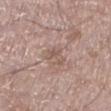No biopsy was performed on this lesion — it was imaged during a full skin examination and was not determined to be concerning. From the left lower leg. The recorded lesion diameter is about 3 mm. The subject is a male in their mid-60s. Automated tile analysis of the lesion measured an area of roughly 4 mm², an outline eccentricity of about 0.5 (0 = round, 1 = elongated), and a symmetry-axis asymmetry near 0.5. The analysis additionally found a mean CIELAB color near L≈55 a*≈17 b*≈24 and a normalized lesion–skin contrast near 5. The analysis additionally found a border-irregularity index near 6/10, a color-variation rating of about 1/10, and peripheral color asymmetry of about 0. A region of skin cropped from a whole-body photographic capture, roughly 15 mm wide. Imaged with white-light lighting.